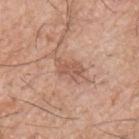* notes — no biopsy performed (imaged during a skin exam)
* body site — the chest
* imaging modality — total-body-photography crop, ~15 mm field of view
* illumination — white-light
* lesion size — ~3.5 mm (longest diameter)
* patient — male, in their mid- to late 70s